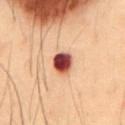This lesion was catalogued during total-body skin photography and was not selected for biopsy. A male subject in their mid- to late 50s. The lesion's longest dimension is about 3 mm. Captured under cross-polarized illumination. From the abdomen. Cropped from a total-body skin-imaging series; the visible field is about 15 mm.